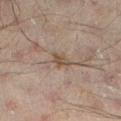Q: Is there a histopathology result?
A: catalogued during a skin exam; not biopsied
Q: Who is the patient?
A: male, aged approximately 45
Q: What is the imaging modality?
A: total-body-photography crop, ~15 mm field of view
Q: What did automated image analysis measure?
A: a border-irregularity index near 3/10, a color-variation rating of about 3/10, and a peripheral color-asymmetry measure near 1; an automated nevus-likeness rating near 0 out of 100
Q: Lesion location?
A: the left lower leg